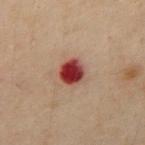| key | value |
|---|---|
| notes | no biopsy performed (imaged during a skin exam) |
| patient | male, roughly 50 years of age |
| lesion diameter | about 3.5 mm |
| imaging modality | 15 mm crop, total-body photography |
| location | the abdomen |
| tile lighting | cross-polarized illumination |